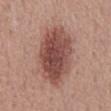Image and clinical context:
Imaged with white-light lighting. Located on the mid back. A 15 mm close-up extracted from a 3D total-body photography capture. A male subject, approximately 55 years of age. Automated tile analysis of the lesion measured an average lesion color of about L≈48 a*≈23 b*≈24 (CIELAB) and about 14 CIELAB-L* units darker than the surrounding skin. It also reported a border-irregularity rating of about 2/10, a color-variation rating of about 5.5/10, and a peripheral color-asymmetry measure near 2. And it measured an automated nevus-likeness rating near 95 out of 100 and lesion-presence confidence of about 100/100. The recorded lesion diameter is about 9 mm.